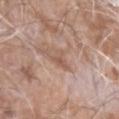Assessment:
This lesion was catalogued during total-body skin photography and was not selected for biopsy.
Context:
Imaged with white-light lighting. This image is a 15 mm lesion crop taken from a total-body photograph. The patient is a male approximately 75 years of age. On the left forearm.